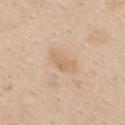| key | value |
|---|---|
| workup | catalogued during a skin exam; not biopsied |
| subject | female, aged around 55 |
| image source | 15 mm crop, total-body photography |
| lesion size | ~3.5 mm (longest diameter) |
| automated lesion analysis | an average lesion color of about L≈66 a*≈15 b*≈34 (CIELAB), a lesion–skin lightness drop of about 7, and a normalized lesion–skin contrast near 5; a classifier nevus-likeness of about 0/100 and a lesion-detection confidence of about 100/100 |
| site | the chest |
| lighting | white-light illumination |